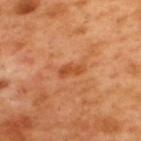Impression:
Recorded during total-body skin imaging; not selected for excision or biopsy.
Clinical summary:
Located on the back. The subject is a male in their 50s. A close-up tile cropped from a whole-body skin photograph, about 15 mm across. Captured under cross-polarized illumination. The recorded lesion diameter is about 3 mm.